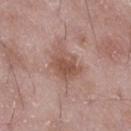<record>
  <patient>
    <sex>male</sex>
    <age_approx>50</age_approx>
  </patient>
  <lighting>white-light</lighting>
  <image>
    <source>total-body photography crop</source>
    <field_of_view_mm>15</field_of_view_mm>
  </image>
  <automated_metrics>
    <cielab_L>52</cielab_L>
    <cielab_a>20</cielab_a>
    <cielab_b>26</cielab_b>
    <vs_skin_darker_L>10.0</vs_skin_darker_L>
    <vs_skin_contrast_norm>7.5</vs_skin_contrast_norm>
    <color_variation_0_10>3.0</color_variation_0_10>
    <peripheral_color_asymmetry>1.0</peripheral_color_asymmetry>
  </automated_metrics>
  <site>right thigh</site>
</record>